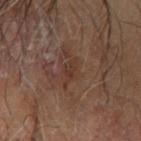Context: The total-body-photography lesion software estimated a lesion color around L≈35 a*≈18 b*≈25 in CIELAB, about 6 CIELAB-L* units darker than the surrounding skin, and a normalized border contrast of about 5.5. And it measured border irregularity of about 3 on a 0–10 scale, internal color variation of about 1.5 on a 0–10 scale, and a peripheral color-asymmetry measure near 0.5. The software also gave lesion-presence confidence of about 95/100. This image is a 15 mm lesion crop taken from a total-body photograph. The lesion's longest dimension is about 3 mm. A male subject approximately 65 years of age. The tile uses cross-polarized illumination. Located on the right forearm.